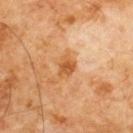biopsy_status: not biopsied; imaged during a skin examination
site: chest
image:
  source: total-body photography crop
  field_of_view_mm: 15
lesion_size:
  long_diameter_mm_approx: 3.0
automated_metrics:
  cielab_L: 54
  cielab_a: 25
  cielab_b: 43
  vs_skin_darker_L: 10.0
  vs_skin_contrast_norm: 8.0
  nevus_likeness_0_100: 5
  lesion_detection_confidence_0_100: 100
patient:
  sex: male
  age_approx: 60
lighting: cross-polarized